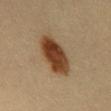{
  "biopsy_status": "not biopsied; imaged during a skin examination"
}